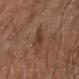notes: imaged on a skin check; not biopsied
size: about 4 mm
lighting: cross-polarized
patient: male, aged approximately 55
body site: the left forearm
image: total-body-photography crop, ~15 mm field of view